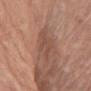Part of a total-body skin-imaging series; this lesion was reviewed on a skin check and was not flagged for biopsy.
The lesion is on the right upper arm.
Imaged with white-light lighting.
A female subject, roughly 65 years of age.
The total-body-photography lesion software estimated a lesion area of about 8.5 mm² and two-axis asymmetry of about 0.3. The analysis additionally found a lesion color around L≈50 a*≈20 b*≈27 in CIELAB, roughly 7 lightness units darker than nearby skin, and a normalized lesion–skin contrast near 5.5. It also reported border irregularity of about 4 on a 0–10 scale, a within-lesion color-variation index near 1.5/10, and a peripheral color-asymmetry measure near 0.5.
This image is a 15 mm lesion crop taken from a total-body photograph.